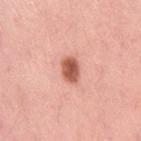Assessment: The lesion was tiled from a total-body skin photograph and was not biopsied. Context: On the right thigh. A roughly 15 mm field-of-view crop from a total-body skin photograph. Longest diameter approximately 3 mm. A female patient aged around 50. Captured under white-light illumination.